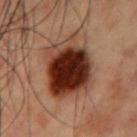Clinical impression:
The lesion was tiled from a total-body skin photograph and was not biopsied.
Clinical summary:
A male subject, about 55 years old. Cropped from a total-body skin-imaging series; the visible field is about 15 mm. On the chest.